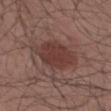Notes:
- notes: imaged on a skin check; not biopsied
- size: about 5 mm
- site: the arm
- subject: male, roughly 50 years of age
- image source: ~15 mm tile from a whole-body skin photo
- tile lighting: white-light illumination
- TBP lesion metrics: a lesion-to-skin contrast of about 7.5 (normalized; higher = more distinct); peripheral color asymmetry of about 1